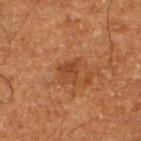subject: male, roughly 65 years of age
image source: 15 mm crop, total-body photography
diameter: about 3 mm
site: the leg